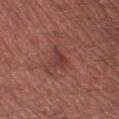follow-up = no biopsy performed (imaged during a skin exam) | size = ≈2.5 mm | illumination = white-light illumination | body site = the left thigh | subject = male, roughly 70 years of age | acquisition = ~15 mm tile from a whole-body skin photo | image-analysis metrics = an area of roughly 3 mm², a shape eccentricity near 0.8, and a symmetry-axis asymmetry near 0.35; border irregularity of about 3.5 on a 0–10 scale, a within-lesion color-variation index near 2.5/10, and peripheral color asymmetry of about 1.